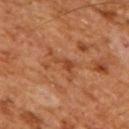follow-up: total-body-photography surveillance lesion; no biopsy
location: the mid back
subject: male, in their mid- to late 60s
automated lesion analysis: a lesion color around L≈44 a*≈25 b*≈35 in CIELAB and a lesion–skin lightness drop of about 7; a border-irregularity rating of about 9/10, internal color variation of about 1 on a 0–10 scale, and peripheral color asymmetry of about 0; an automated nevus-likeness rating near 0 out of 100 and a lesion-detection confidence of about 100/100
acquisition: 15 mm crop, total-body photography